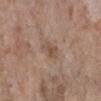follow-up=total-body-photography surveillance lesion; no biopsy
subject=female, approximately 85 years of age or older
body site=the right lower leg
image=~15 mm crop, total-body skin-cancer survey
size=~2.5 mm (longest diameter)
tile lighting=white-light illumination